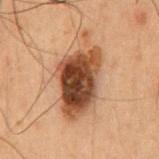Clinical impression: The lesion was photographed on a routine skin check and not biopsied; there is no pathology result. Image and clinical context: The recorded lesion diameter is about 9 mm. A roughly 15 mm field-of-view crop from a total-body skin photograph. The lesion is on the abdomen. A male patient, approximately 50 years of age. Imaged with cross-polarized lighting.